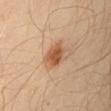Q: Was a biopsy performed?
A: imaged on a skin check; not biopsied
Q: What lighting was used for the tile?
A: cross-polarized illumination
Q: What is the imaging modality?
A: ~15 mm crop, total-body skin-cancer survey
Q: Where on the body is the lesion?
A: the left upper arm
Q: What did automated image analysis measure?
A: an area of roughly 6 mm², a shape eccentricity near 0.55, and a symmetry-axis asymmetry near 0.15; a border-irregularity rating of about 1.5/10 and a within-lesion color-variation index near 3/10; a classifier nevus-likeness of about 100/100 and a detector confidence of about 100 out of 100 that the crop contains a lesion
Q: Who is the patient?
A: male, in their 30s
Q: How large is the lesion?
A: ≈3 mm